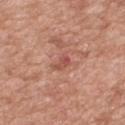A 15 mm close-up extracted from a 3D total-body photography capture.
From the mid back.
Captured under white-light illumination.
A male patient about 50 years old.
Approximately 2.5 mm at its widest.